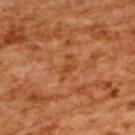Clinical impression: Captured during whole-body skin photography for melanoma surveillance; the lesion was not biopsied. Background: This is a cross-polarized tile. From the upper back. A region of skin cropped from a whole-body photographic capture, roughly 15 mm wide. A female subject roughly 55 years of age. Approximately 3 mm at its widest. Automated tile analysis of the lesion measured an eccentricity of roughly 0.9 and a shape-asymmetry score of about 0.45 (0 = symmetric). The analysis additionally found border irregularity of about 4.5 on a 0–10 scale, a color-variation rating of about 1.5/10, and a peripheral color-asymmetry measure near 0.5. The analysis additionally found a classifier nevus-likeness of about 0/100 and lesion-presence confidence of about 100/100.